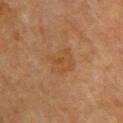Clinical impression: The lesion was tiled from a total-body skin photograph and was not biopsied. Clinical summary: The recorded lesion diameter is about 3.5 mm. The subject is a female about 70 years old. A 15 mm crop from a total-body photograph taken for skin-cancer surveillance. On the upper back. The tile uses cross-polarized illumination. Automated tile analysis of the lesion measured a shape eccentricity near 0.45. The analysis additionally found a lesion color around L≈42 a*≈18 b*≈33 in CIELAB and roughly 5 lightness units darker than nearby skin.